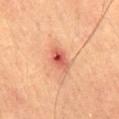This lesion was catalogued during total-body skin photography and was not selected for biopsy. This image is a 15 mm lesion crop taken from a total-body photograph. The lesion is located on the lower back. The subject is a male in their mid-60s. Longest diameter approximately 3 mm. This is a cross-polarized tile.